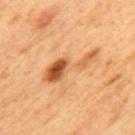Acquisition and patient details:
Captured under cross-polarized illumination. A male subject in their 50s. Longest diameter approximately 7 mm. Cropped from a total-body skin-imaging series; the visible field is about 15 mm. The lesion is located on the mid back.